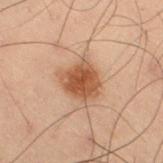{
  "biopsy_status": "not biopsied; imaged during a skin examination",
  "lighting": "cross-polarized",
  "patient": {
    "sex": "male",
    "age_approx": 55
  },
  "image": {
    "source": "total-body photography crop",
    "field_of_view_mm": 15
  },
  "automated_metrics": {
    "eccentricity": 0.5,
    "cielab_L": 43,
    "cielab_a": 19,
    "cielab_b": 29,
    "vs_skin_darker_L": 11.0,
    "border_irregularity_0_10": 2.5,
    "color_variation_0_10": 3.5,
    "peripheral_color_asymmetry": 1.0
  },
  "lesion_size": {
    "long_diameter_mm_approx": 4.0
  },
  "site": "right thigh"
}